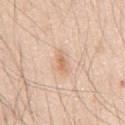Clinical impression: This lesion was catalogued during total-body skin photography and was not selected for biopsy. Acquisition and patient details: A roughly 15 mm field-of-view crop from a total-body skin photograph. A male subject, roughly 45 years of age. Located on the front of the torso.